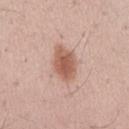Findings:
- biopsy status: imaged on a skin check; not biopsied
- image: 15 mm crop, total-body photography
- subject: male, aged 33–37
- anatomic site: the mid back